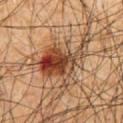Q: What kind of image is this?
A: total-body-photography crop, ~15 mm field of view
Q: Automated lesion metrics?
A: border irregularity of about 8.5 on a 0–10 scale and a color-variation rating of about 10/10
Q: What are the patient's age and sex?
A: male, aged approximately 65
Q: What lighting was used for the tile?
A: cross-polarized
Q: Lesion location?
A: the chest
Q: How large is the lesion?
A: ≈8.5 mm